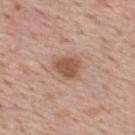The lesion was tiled from a total-body skin photograph and was not biopsied. The lesion is on the mid back. The subject is a male aged 73 to 77. Approximately 3.5 mm at its widest. Captured under white-light illumination. A lesion tile, about 15 mm wide, cut from a 3D total-body photograph. An algorithmic analysis of the crop reported an area of roughly 8 mm², an outline eccentricity of about 0.65 (0 = round, 1 = elongated), and a shape-asymmetry score of about 0.25 (0 = symmetric). It also reported a border-irregularity index near 2.5/10, a within-lesion color-variation index near 2/10, and peripheral color asymmetry of about 0.5.